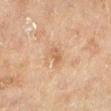Captured during whole-body skin photography for melanoma surveillance; the lesion was not biopsied. A 15 mm crop from a total-body photograph taken for skin-cancer surveillance. A female subject, in their mid-50s. Automated image analysis of the tile measured a footprint of about 3.5 mm², an eccentricity of roughly 0.8, and two-axis asymmetry of about 0.3. It also reported a lesion color around L≈53 a*≈18 b*≈32 in CIELAB and about 7 CIELAB-L* units darker than the surrounding skin. The software also gave a detector confidence of about 100 out of 100 that the crop contains a lesion. Measured at roughly 2.5 mm in maximum diameter. On the leg.